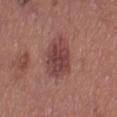The lesion was tiled from a total-body skin photograph and was not biopsied.
The lesion is on the right thigh.
Approximately 5.5 mm at its widest.
A lesion tile, about 15 mm wide, cut from a 3D total-body photograph.
Automated image analysis of the tile measured border irregularity of about 2 on a 0–10 scale, internal color variation of about 3.5 on a 0–10 scale, and peripheral color asymmetry of about 1.5. The software also gave a nevus-likeness score of about 90/100 and lesion-presence confidence of about 100/100.
The tile uses white-light illumination.
A female subject, in their 40s.